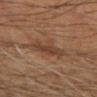Clinical impression: No biopsy was performed on this lesion — it was imaged during a full skin examination and was not determined to be concerning. Image and clinical context: Measured at roughly 4.5 mm in maximum diameter. A close-up tile cropped from a whole-body skin photograph, about 15 mm across. From the left forearm. Automated image analysis of the tile measured an area of roughly 9.5 mm², an eccentricity of roughly 0.8, and a shape-asymmetry score of about 0.4 (0 = symmetric). And it measured a border-irregularity index near 5.5/10, internal color variation of about 2.5 on a 0–10 scale, and a peripheral color-asymmetry measure near 1. It also reported an automated nevus-likeness rating near 0 out of 100 and lesion-presence confidence of about 90/100. A male subject, aged 68 to 72.